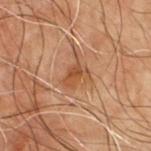Notes:
– tile lighting: cross-polarized
– acquisition: ~15 mm tile from a whole-body skin photo
– patient: male, approximately 70 years of age
– anatomic site: the chest
– diameter: about 3 mm
– TBP lesion metrics: border irregularity of about 3.5 on a 0–10 scale, a within-lesion color-variation index near 3/10, and a peripheral color-asymmetry measure near 1